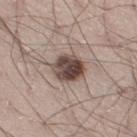Measured at roughly 3.5 mm in maximum diameter. From the right thigh. Cropped from a whole-body photographic skin survey; the tile spans about 15 mm. The lesion-visualizer software estimated a footprint of about 11 mm², an eccentricity of roughly 0.25, and two-axis asymmetry of about 0.15. The software also gave an average lesion color of about L≈46 a*≈15 b*≈20 (CIELAB) and a normalized border contrast of about 12. It also reported a border-irregularity rating of about 1.5/10 and a within-lesion color-variation index near 7.5/10. The analysis additionally found a classifier nevus-likeness of about 50/100. A male patient, roughly 25 years of age.